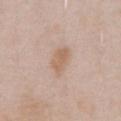Assessment: This lesion was catalogued during total-body skin photography and was not selected for biopsy. Image and clinical context: On the abdomen. A male patient in their mid- to late 50s. A 15 mm close-up extracted from a 3D total-body photography capture.